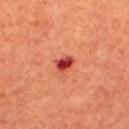Clinical summary: A region of skin cropped from a whole-body photographic capture, roughly 15 mm wide. A male patient, about 65 years old. The lesion is located on the chest. Measured at roughly 2.5 mm in maximum diameter. Automated tile analysis of the lesion measured a mean CIELAB color near L≈43 a*≈38 b*≈32 and a lesion-to-skin contrast of about 11 (normalized; higher = more distinct). It also reported a within-lesion color-variation index near 6.5/10 and peripheral color asymmetry of about 2.5. It also reported a classifier nevus-likeness of about 0/100 and a lesion-detection confidence of about 100/100. Captured under cross-polarized illumination.